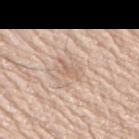  biopsy_status: not biopsied; imaged during a skin examination
  site: right upper arm
  patient:
    sex: male
    age_approx: 75
  lesion_size:
    long_diameter_mm_approx: 2.5
  image:
    source: total-body photography crop
    field_of_view_mm: 15
  lighting: white-light